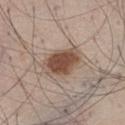Imaged during a routine full-body skin examination; the lesion was not biopsied and no histopathology is available. Longest diameter approximately 5.5 mm. The total-body-photography lesion software estimated an area of roughly 12 mm², an outline eccentricity of about 0.85 (0 = round, 1 = elongated), and two-axis asymmetry of about 0.15. The software also gave a border-irregularity rating of about 2/10 and a within-lesion color-variation index near 4/10. The lesion is located on the leg. Captured under white-light illumination. A male subject, aged 43–47. Cropped from a total-body skin-imaging series; the visible field is about 15 mm.